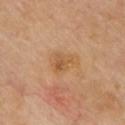Impression: Part of a total-body skin-imaging series; this lesion was reviewed on a skin check and was not flagged for biopsy. Clinical summary: The lesion-visualizer software estimated a footprint of about 7.5 mm², an eccentricity of roughly 0.7, and two-axis asymmetry of about 0.4. It also reported a lesion color around L≈58 a*≈20 b*≈39 in CIELAB, roughly 7 lightness units darker than nearby skin, and a lesion-to-skin contrast of about 6 (normalized; higher = more distinct). The software also gave an automated nevus-likeness rating near 25 out of 100 and lesion-presence confidence of about 100/100. From the chest. Longest diameter approximately 3.5 mm. A lesion tile, about 15 mm wide, cut from a 3D total-body photograph. The tile uses cross-polarized illumination.